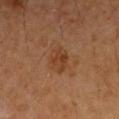Clinical summary: Cropped from a whole-body photographic skin survey; the tile spans about 15 mm. On the right upper arm. The tile uses cross-polarized illumination. The lesion's longest dimension is about 3.5 mm. The subject is a male aged 58–62.